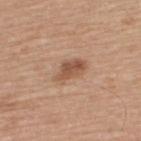The lesion was photographed on a routine skin check and not biopsied; there is no pathology result.
The lesion is on the back.
This is a white-light tile.
Measured at roughly 4 mm in maximum diameter.
The patient is a male in their mid-60s.
A region of skin cropped from a whole-body photographic capture, roughly 15 mm wide.
An algorithmic analysis of the crop reported an area of roughly 6.5 mm² and a symmetry-axis asymmetry near 0.3. The analysis additionally found border irregularity of about 3 on a 0–10 scale, internal color variation of about 3.5 on a 0–10 scale, and radial color variation of about 1.5.